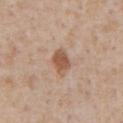notes: total-body-photography surveillance lesion; no biopsy | subject: male, aged 63 to 67 | automated metrics: an area of roughly 5.5 mm², an outline eccentricity of about 0.7 (0 = round, 1 = elongated), and a shape-asymmetry score of about 0.3 (0 = symmetric); a mean CIELAB color near L≈55 a*≈20 b*≈31 and a normalized border contrast of about 8.5; a classifier nevus-likeness of about 80/100 and a detector confidence of about 100 out of 100 that the crop contains a lesion | lighting: white-light | acquisition: ~15 mm crop, total-body skin-cancer survey | lesion diameter: about 3 mm | anatomic site: the chest.